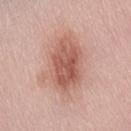Imaged during a routine full-body skin examination; the lesion was not biopsied and no histopathology is available. A female patient aged around 55. Located on the lower back. A 15 mm crop from a total-body photograph taken for skin-cancer surveillance.